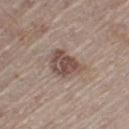<record>
  <biopsy_status>not biopsied; imaged during a skin examination</biopsy_status>
  <image>
    <source>total-body photography crop</source>
    <field_of_view_mm>15</field_of_view_mm>
  </image>
  <lighting>white-light</lighting>
  <automated_metrics>
    <eccentricity>0.7</eccentricity>
    <shape_asymmetry>0.3</shape_asymmetry>
    <vs_skin_darker_L>12.0</vs_skin_darker_L>
  </automated_metrics>
  <patient>
    <sex>male</sex>
    <age_approx>65</age_approx>
  </patient>
  <site>left thigh</site>
</record>